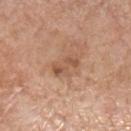This lesion was catalogued during total-body skin photography and was not selected for biopsy.